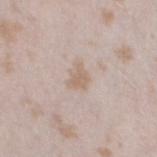Clinical impression: Captured during whole-body skin photography for melanoma surveillance; the lesion was not biopsied. Clinical summary: The tile uses white-light illumination. Automated tile analysis of the lesion measured an area of roughly 3.5 mm², an eccentricity of roughly 0.65, and a shape-asymmetry score of about 0.45 (0 = symmetric). It also reported a border-irregularity index near 4.5/10, a color-variation rating of about 1/10, and radial color variation of about 0.5. A 15 mm crop from a total-body photograph taken for skin-cancer surveillance. The recorded lesion diameter is about 2.5 mm. The patient is a female aged around 25. From the leg.